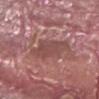This lesion was catalogued during total-body skin photography and was not selected for biopsy.